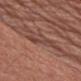  biopsy_status: not biopsied; imaged during a skin examination
  patient:
    sex: male
    age_approx: 70
  lesion_size:
    long_diameter_mm_approx: 4.5
  automated_metrics:
    border_irregularity_0_10: 4.0
    color_variation_0_10: 5.0
    peripheral_color_asymmetry: 1.5
  lighting: white-light
  site: front of the torso
  image:
    source: total-body photography crop
    field_of_view_mm: 15